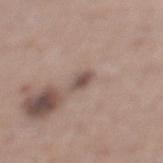Captured during whole-body skin photography for melanoma surveillance; the lesion was not biopsied. A lesion tile, about 15 mm wide, cut from a 3D total-body photograph. On the left lower leg. The subject is a male aged 63 to 67. Captured under white-light illumination. An algorithmic analysis of the crop reported lesion-presence confidence of about 100/100. Approximately 2.5 mm at its widest.